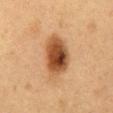Imaged during a routine full-body skin examination; the lesion was not biopsied and no histopathology is available. Longest diameter approximately 5.5 mm. A male subject in their mid- to late 50s. A 15 mm crop from a total-body photograph taken for skin-cancer surveillance. This is a cross-polarized tile. Located on the abdomen.